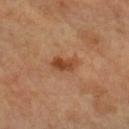notes: total-body-photography surveillance lesion; no biopsy | lighting: cross-polarized illumination | patient: female, in their 60s | lesion size: ~3 mm (longest diameter) | image: 15 mm crop, total-body photography | automated metrics: a footprint of about 5 mm² and an outline eccentricity of about 0.8 (0 = round, 1 = elongated); an average lesion color of about L≈47 a*≈25 b*≈36 (CIELAB) and a lesion-to-skin contrast of about 8.5 (normalized; higher = more distinct) | anatomic site: the left forearm.